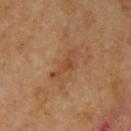Notes:
* follow-up · imaged on a skin check; not biopsied
* patient · female, aged 68 to 72
* tile lighting · cross-polarized illumination
* diameter · about 4 mm
* location · the arm
* image-analysis metrics · a mean CIELAB color near L≈39 a*≈20 b*≈30, a lesion–skin lightness drop of about 6, and a normalized border contrast of about 5.5; a border-irregularity index near 7/10 and a peripheral color-asymmetry measure near 0; an automated nevus-likeness rating near 0 out of 100 and a lesion-detection confidence of about 100/100
* image source · ~15 mm crop, total-body skin-cancer survey